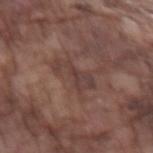– notes · imaged on a skin check; not biopsied
– image source · total-body-photography crop, ~15 mm field of view
– patient · male, in their mid-70s
– lighting · white-light
– diameter · ~4.5 mm (longest diameter)
– site · the left forearm